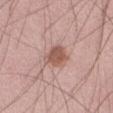biopsy status: catalogued during a skin exam; not biopsied
imaging modality: 15 mm crop, total-body photography
body site: the abdomen
tile lighting: white-light
subject: male, roughly 75 years of age
image-analysis metrics: a lesion color around L≈55 a*≈21 b*≈25 in CIELAB and a lesion-to-skin contrast of about 8 (normalized; higher = more distinct); a border-irregularity index near 2.5/10, internal color variation of about 2.5 on a 0–10 scale, and a peripheral color-asymmetry measure near 0.5